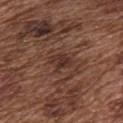Case summary:
* image · ~15 mm tile from a whole-body skin photo
* subject · male, aged 73–77
* site · the chest
* size · ≈3 mm
* tile lighting · white-light